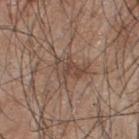| feature | finding |
|---|---|
| follow-up | no biopsy performed (imaged during a skin exam) |
| site | the upper back |
| automated lesion analysis | a lesion area of about 7.5 mm² and a shape-asymmetry score of about 0.45 (0 = symmetric); about 8 CIELAB-L* units darker than the surrounding skin and a lesion-to-skin contrast of about 6.5 (normalized; higher = more distinct); border irregularity of about 5 on a 0–10 scale and a within-lesion color-variation index near 3.5/10 |
| image source | ~15 mm crop, total-body skin-cancer survey |
| patient | male, aged approximately 45 |
| illumination | white-light illumination |
| lesion size | ≈3.5 mm |